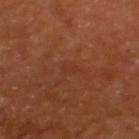Recorded during total-body skin imaging; not selected for excision or biopsy.
Captured under cross-polarized illumination.
The patient is a female aged 48–52.
About 2 mm across.
Located on the upper back.
A roughly 15 mm field-of-view crop from a total-body skin photograph.